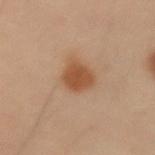A region of skin cropped from a whole-body photographic capture, roughly 15 mm wide. From the left upper arm. A male patient, aged approximately 55. The lesion's longest dimension is about 3.5 mm. Captured under cross-polarized illumination.